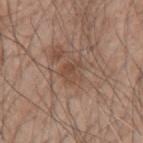follow-up: imaged on a skin check; not biopsied | tile lighting: white-light illumination | patient: male, in their 50s | lesion diameter: about 2.5 mm | site: the left upper arm | image: 15 mm crop, total-body photography.